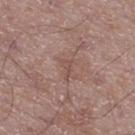This is a white-light tile.
The lesion's longest dimension is about 2.5 mm.
A male patient roughly 50 years of age.
A 15 mm close-up extracted from a 3D total-body photography capture.
Located on the right thigh.
The total-body-photography lesion software estimated border irregularity of about 5 on a 0–10 scale, a within-lesion color-variation index near 0/10, and peripheral color asymmetry of about 0.